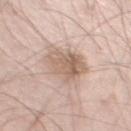Captured during whole-body skin photography for melanoma surveillance; the lesion was not biopsied. The recorded lesion diameter is about 4.5 mm. The total-body-photography lesion software estimated a shape-asymmetry score of about 0.25 (0 = symmetric). And it measured border irregularity of about 3 on a 0–10 scale, a within-lesion color-variation index near 5.5/10, and radial color variation of about 2. The software also gave a nevus-likeness score of about 20/100. From the left thigh. Cropped from a total-body skin-imaging series; the visible field is about 15 mm. The tile uses white-light illumination. The patient is a male in their 60s.